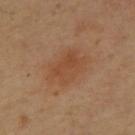Part of a total-body skin-imaging series; this lesion was reviewed on a skin check and was not flagged for biopsy.
A male subject about 55 years old.
A 15 mm close-up tile from a total-body photography series done for melanoma screening.
Longest diameter approximately 5 mm.
The lesion is located on the upper back.
The total-body-photography lesion software estimated internal color variation of about 3 on a 0–10 scale and peripheral color asymmetry of about 1. The software also gave a classifier nevus-likeness of about 70/100 and a detector confidence of about 100 out of 100 that the crop contains a lesion.